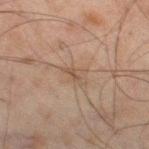notes: catalogued during a skin exam; not biopsied
subject: male, about 45 years old
imaging modality: ~15 mm crop, total-body skin-cancer survey
site: the right thigh
lighting: cross-polarized illumination